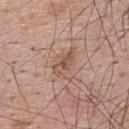<tbp_lesion>
  <image>
    <source>total-body photography crop</source>
    <field_of_view_mm>15</field_of_view_mm>
  </image>
  <patient>
    <sex>male</sex>
    <age_approx>65</age_approx>
  </patient>
  <lesion_size>
    <long_diameter_mm_approx>4.0</long_diameter_mm_approx>
  </lesion_size>
  <site>upper back</site>
  <lighting>white-light</lighting>
</tbp_lesion>